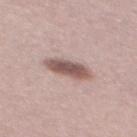Case summary:
* subject · male, approximately 30 years of age
* acquisition · 15 mm crop, total-body photography